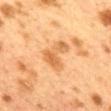biopsy_status: not biopsied; imaged during a skin examination
site: mid back
image:
  source: total-body photography crop
  field_of_view_mm: 15
patient:
  sex: female
  age_approx: 40
automated_metrics:
  area_mm2_approx: 8.0
  eccentricity: 0.8
  cielab_L: 54
  cielab_a: 21
  cielab_b: 38
  vs_skin_contrast_norm: 6.5
  nevus_likeness_0_100: 50
  lesion_detection_confidence_0_100: 100